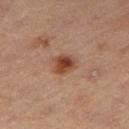Assessment:
The lesion was tiled from a total-body skin photograph and was not biopsied.
Clinical summary:
This is a cross-polarized tile. From the right thigh. A male subject aged approximately 55. A 15 mm close-up tile from a total-body photography series done for melanoma screening. Approximately 3 mm at its widest.